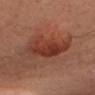Case summary:
* notes — imaged on a skin check; not biopsied
* location — the head or neck
* tile lighting — cross-polarized illumination
* subject — female, about 45 years old
* lesion size — ~6 mm (longest diameter)
* acquisition — ~15 mm tile from a whole-body skin photo